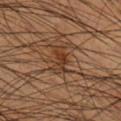Acquisition and patient details:
The lesion is on the leg. Longest diameter approximately 3.5 mm. A male subject, aged 43 to 47. A roughly 15 mm field-of-view crop from a total-body skin photograph. This is a cross-polarized tile. Automated tile analysis of the lesion measured a lesion area of about 6 mm², a shape eccentricity near 0.85, and a symmetry-axis asymmetry near 0.4. The analysis additionally found border irregularity of about 5 on a 0–10 scale, a within-lesion color-variation index near 6.5/10, and peripheral color asymmetry of about 2. The analysis additionally found a classifier nevus-likeness of about 0/100 and a lesion-detection confidence of about 95/100.